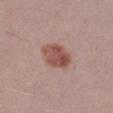workup: total-body-photography surveillance lesion; no biopsy
lesion diameter: about 4.5 mm
patient: female, aged approximately 25
body site: the leg
image source: 15 mm crop, total-body photography